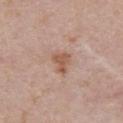Assessment:
Captured during whole-body skin photography for melanoma surveillance; the lesion was not biopsied.
Background:
The lesion is located on the chest. A female patient, about 50 years old. A region of skin cropped from a whole-body photographic capture, roughly 15 mm wide.